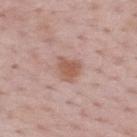Q: Was a biopsy performed?
A: imaged on a skin check; not biopsied
Q: Illumination type?
A: white-light
Q: What did automated image analysis measure?
A: an area of roughly 5.5 mm², an outline eccentricity of about 0.5 (0 = round, 1 = elongated), and a shape-asymmetry score of about 0.35 (0 = symmetric); a lesion–skin lightness drop of about 10 and a normalized border contrast of about 8; a border-irregularity rating of about 3/10, a within-lesion color-variation index near 2/10, and radial color variation of about 0.5
Q: Who is the patient?
A: male, aged around 50
Q: What kind of image is this?
A: total-body-photography crop, ~15 mm field of view
Q: What is the anatomic site?
A: the back